A male patient roughly 50 years of age; from the head or neck; cropped from a total-body skin-imaging series; the visible field is about 15 mm: 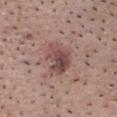Longest diameter approximately 4 mm. Automated image analysis of the tile measured an area of roughly 10 mm² and a symmetry-axis asymmetry near 0.25. It also reported about 11 CIELAB-L* units darker than the surrounding skin and a normalized border contrast of about 8. It also reported border irregularity of about 3 on a 0–10 scale, a color-variation rating of about 7/10, and radial color variation of about 2.5. The tile uses white-light illumination. Histopathologically confirmed as a seborrheic keratosis.